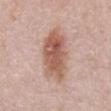Imaged during a routine full-body skin examination; the lesion was not biopsied and no histopathology is available.
From the abdomen.
A close-up tile cropped from a whole-body skin photograph, about 15 mm across.
A male patient aged 78 to 82.
The lesion-visualizer software estimated a shape eccentricity near 0.85 and a symmetry-axis asymmetry near 0.2. The analysis additionally found internal color variation of about 6 on a 0–10 scale and a peripheral color-asymmetry measure near 1.5. It also reported a classifier nevus-likeness of about 95/100.
Measured at roughly 7 mm in maximum diameter.